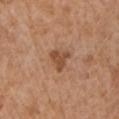Q: Was a biopsy performed?
A: catalogued during a skin exam; not biopsied
Q: Who is the patient?
A: male, about 65 years old
Q: What kind of image is this?
A: ~15 mm tile from a whole-body skin photo
Q: Lesion location?
A: the left upper arm